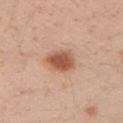Recorded during total-body skin imaging; not selected for excision or biopsy. A male subject, in their 30s. A 15 mm crop from a total-body photograph taken for skin-cancer surveillance. The lesion is on the left upper arm.